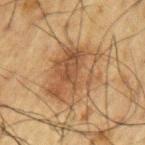notes: no biopsy performed (imaged during a skin exam)
image source: ~15 mm crop, total-body skin-cancer survey
illumination: cross-polarized illumination
patient: male, about 60 years old
lesion diameter: ~7 mm (longest diameter)
TBP lesion metrics: an area of roughly 25 mm², a shape eccentricity near 0.7, and a shape-asymmetry score of about 0.25 (0 = symmetric); an average lesion color of about L≈40 a*≈16 b*≈29 (CIELAB), roughly 8 lightness units darker than nearby skin, and a lesion-to-skin contrast of about 7 (normalized; higher = more distinct); a border-irregularity index near 3.5/10 and radial color variation of about 2
location: the left upper arm